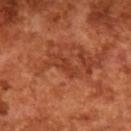{"image": {"source": "total-body photography crop", "field_of_view_mm": 15}, "patient": {"sex": "male", "age_approx": 65}}